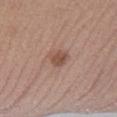notes=catalogued during a skin exam; not biopsied
imaging modality=15 mm crop, total-body photography
patient=female, roughly 40 years of age
body site=the right lower leg